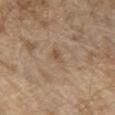Located on the chest. A male subject aged approximately 70. Automated tile analysis of the lesion measured an average lesion color of about L≈53 a*≈15 b*≈30 (CIELAB). The analysis additionally found internal color variation of about 2.5 on a 0–10 scale and peripheral color asymmetry of about 1. The analysis additionally found a nevus-likeness score of about 0/100 and a lesion-detection confidence of about 100/100. A roughly 15 mm field-of-view crop from a total-body skin photograph. The tile uses white-light illumination. About 3.5 mm across.